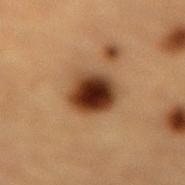Q: Was a biopsy performed?
A: imaged on a skin check; not biopsied
Q: What is the anatomic site?
A: the back
Q: What kind of image is this?
A: 15 mm crop, total-body photography
Q: What lighting was used for the tile?
A: cross-polarized
Q: Lesion size?
A: about 4 mm
Q: What are the patient's age and sex?
A: male, aged 83 or older